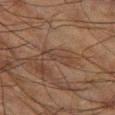Assessment: The lesion was photographed on a routine skin check and not biopsied; there is no pathology result. Context: A male patient aged around 65. This image is a 15 mm lesion crop taken from a total-body photograph. From the left thigh.